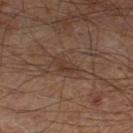biopsy status: no biopsy performed (imaged during a skin exam)
acquisition: ~15 mm tile from a whole-body skin photo
patient: male, aged 58 to 62
lighting: cross-polarized
automated metrics: an eccentricity of roughly 0.9 and a symmetry-axis asymmetry near 0.3; an automated nevus-likeness rating near 0 out of 100 and a detector confidence of about 95 out of 100 that the crop contains a lesion
location: the leg
lesion size: ≈3.5 mm